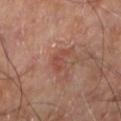Part of a total-body skin-imaging series; this lesion was reviewed on a skin check and was not flagged for biopsy.
A roughly 15 mm field-of-view crop from a total-body skin photograph.
This is a cross-polarized tile.
A male subject approximately 70 years of age.
The recorded lesion diameter is about 3 mm.
The lesion is located on the left lower leg.
The lesion-visualizer software estimated a lesion color around L≈46 a*≈25 b*≈27 in CIELAB, about 7 CIELAB-L* units darker than the surrounding skin, and a normalized border contrast of about 5.5. And it measured a border-irregularity index near 4.5/10, a within-lesion color-variation index near 1.5/10, and a peripheral color-asymmetry measure near 0.5. And it measured a lesion-detection confidence of about 100/100.